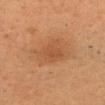Assessment: This lesion was catalogued during total-body skin photography and was not selected for biopsy. Context: A male subject, aged 38 to 42. Cropped from a whole-body photographic skin survey; the tile spans about 15 mm. From the head or neck. The tile uses cross-polarized illumination.